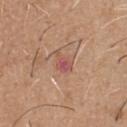notes: no biopsy performed (imaged during a skin exam) | location: the chest | patient: male, approximately 65 years of age | lighting: white-light illumination | lesion size: about 3 mm | image source: 15 mm crop, total-body photography.